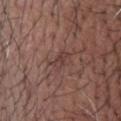follow-up=no biopsy performed (imaged during a skin exam)
location=the head or neck
automated metrics=a shape eccentricity near 0.85 and two-axis asymmetry of about 0.4; border irregularity of about 5 on a 0–10 scale, a color-variation rating of about 1.5/10, and radial color variation of about 0.5
diameter=≈3.5 mm
lighting=white-light illumination
image source=~15 mm tile from a whole-body skin photo
subject=male, aged around 55